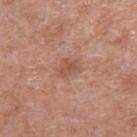This lesion was catalogued during total-body skin photography and was not selected for biopsy. This is a white-light tile. The subject is a male in their 70s. Measured at roughly 3 mm in maximum diameter. The lesion is located on the right upper arm. This image is a 15 mm lesion crop taken from a total-body photograph.